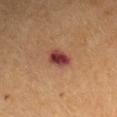<lesion>
  <biopsy_status>not biopsied; imaged during a skin examination</biopsy_status>
  <image>
    <source>total-body photography crop</source>
    <field_of_view_mm>15</field_of_view_mm>
  </image>
  <patient>
    <sex>male</sex>
    <age_approx>65</age_approx>
  </patient>
  <site>left upper arm</site>
</lesion>